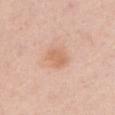No biopsy was performed on this lesion — it was imaged during a full skin examination and was not determined to be concerning. The tile uses white-light illumination. The lesion is located on the chest. The patient is a female aged 48 to 52. An algorithmic analysis of the crop reported a lesion area of about 6 mm², a shape eccentricity near 0.55, and a symmetry-axis asymmetry near 0.2. The analysis additionally found an average lesion color of about L≈66 a*≈22 b*≈33 (CIELAB), roughly 8 lightness units darker than nearby skin, and a normalized border contrast of about 6. The software also gave a within-lesion color-variation index near 2/10 and radial color variation of about 1. And it measured lesion-presence confidence of about 100/100. A 15 mm close-up extracted from a 3D total-body photography capture.